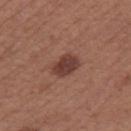Notes:
- follow-up — no biopsy performed (imaged during a skin exam)
- patient — female, aged 38–42
- lighting — white-light illumination
- image — ~15 mm crop, total-body skin-cancer survey
- automated metrics — a shape-asymmetry score of about 0.25 (0 = symmetric); an average lesion color of about L≈39 a*≈22 b*≈24 (CIELAB), roughly 11 lightness units darker than nearby skin, and a normalized lesion–skin contrast near 9; a border-irregularity rating of about 2.5/10, a color-variation rating of about 3/10, and radial color variation of about 1
- anatomic site — the leg
- lesion size — ~3.5 mm (longest diameter)